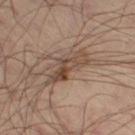Findings:
* biopsy status — catalogued during a skin exam; not biopsied
* patient — male, about 50 years old
* image source — ~15 mm tile from a whole-body skin photo
* lesion size — about 5.5 mm
* tile lighting — cross-polarized illumination
* TBP lesion metrics — a lesion area of about 7 mm², an eccentricity of roughly 0.95, and a shape-asymmetry score of about 0.5 (0 = symmetric); a lesion color around L≈44 a*≈16 b*≈26 in CIELAB and a lesion–skin lightness drop of about 11; an automated nevus-likeness rating near 50 out of 100 and a detector confidence of about 100 out of 100 that the crop contains a lesion
* body site — the abdomen